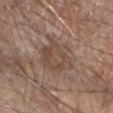{
  "biopsy_status": "not biopsied; imaged during a skin examination",
  "lighting": "white-light",
  "image": {
    "source": "total-body photography crop",
    "field_of_view_mm": 15
  },
  "lesion_size": {
    "long_diameter_mm_approx": 5.0
  },
  "patient": {
    "sex": "male",
    "age_approx": 80
  },
  "site": "right forearm"
}